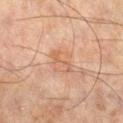Recorded during total-body skin imaging; not selected for excision or biopsy. Located on the right lower leg. The patient is a male aged 43–47. A close-up tile cropped from a whole-body skin photograph, about 15 mm across. Captured under cross-polarized illumination. The total-body-photography lesion software estimated a footprint of about 7 mm², an outline eccentricity of about 0.6 (0 = round, 1 = elongated), and a symmetry-axis asymmetry near 0.3. The analysis additionally found a color-variation rating of about 4/10 and radial color variation of about 1.5.